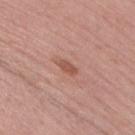Impression: Recorded during total-body skin imaging; not selected for excision or biopsy. Acquisition and patient details: A 15 mm crop from a total-body photograph taken for skin-cancer surveillance. Measured at roughly 3 mm in maximum diameter. A female patient, approximately 60 years of age. The lesion is located on the leg. Imaged with white-light lighting. The total-body-photography lesion software estimated an automated nevus-likeness rating near 20 out of 100.